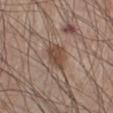workup=no biopsy performed (imaged during a skin exam) | tile lighting=white-light | patient=male, in their 60s | acquisition=~15 mm tile from a whole-body skin photo | automated metrics=an automated nevus-likeness rating near 55 out of 100 | anatomic site=the right lower leg.